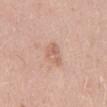TBP lesion metrics: an area of roughly 4.5 mm², a shape eccentricity near 0.85, and a shape-asymmetry score of about 0.45 (0 = symmetric); a border-irregularity rating of about 4.5/10, a color-variation rating of about 2.5/10, and peripheral color asymmetry of about 0.5; a nevus-likeness score of about 0/100 and lesion-presence confidence of about 100/100 | lesion diameter: ~3.5 mm (longest diameter) | image source: ~15 mm tile from a whole-body skin photo | body site: the back | subject: male, about 35 years old.